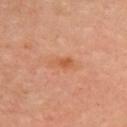Impression:
The lesion was tiled from a total-body skin photograph and was not biopsied.
Acquisition and patient details:
The subject is a female in their 60s. A roughly 15 mm field-of-view crop from a total-body skin photograph. The lesion is on the upper back. This is a cross-polarized tile. The recorded lesion diameter is about 3.5 mm.